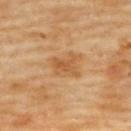Notes:
* workup — catalogued during a skin exam; not biopsied